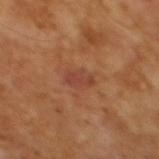Imaged during a routine full-body skin examination; the lesion was not biopsied and no histopathology is available.
A male patient, aged 63–67.
A close-up tile cropped from a whole-body skin photograph, about 15 mm across.